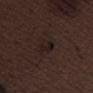<case>
<biopsy_status>not biopsied; imaged during a skin examination</biopsy_status>
<lighting>white-light</lighting>
<patient>
  <sex>male</sex>
  <age_approx>70</age_approx>
</patient>
<site>left thigh</site>
<image>
  <source>total-body photography crop</source>
  <field_of_view_mm>15</field_of_view_mm>
</image>
<lesion_size>
  <long_diameter_mm_approx>2.5</long_diameter_mm_approx>
</lesion_size>
</case>